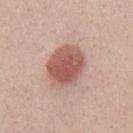Impression:
Part of a total-body skin-imaging series; this lesion was reviewed on a skin check and was not flagged for biopsy.
Context:
The tile uses white-light illumination. Cropped from a whole-body photographic skin survey; the tile spans about 15 mm. From the mid back. A male patient aged 38 to 42.